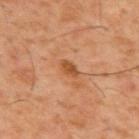Part of a total-body skin-imaging series; this lesion was reviewed on a skin check and was not flagged for biopsy. The lesion-visualizer software estimated an outline eccentricity of about 0.8 (0 = round, 1 = elongated) and a shape-asymmetry score of about 0.2 (0 = symmetric). And it measured an automated nevus-likeness rating near 10 out of 100 and a lesion-detection confidence of about 100/100. The subject is a male aged around 60. On the upper back. A close-up tile cropped from a whole-body skin photograph, about 15 mm across.